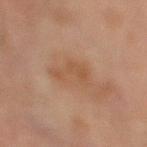workup: imaged on a skin check; not biopsied | subject: female, roughly 70 years of age | lesion size: about 5 mm | acquisition: ~15 mm tile from a whole-body skin photo | site: the leg.